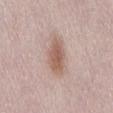notes — total-body-photography surveillance lesion; no biopsy
patient — female, aged 48–52
image-analysis metrics — a lesion area of about 11 mm², an eccentricity of roughly 0.9, and a symmetry-axis asymmetry near 0.15
lesion diameter — ≈5.5 mm
site — the back
image — ~15 mm tile from a whole-body skin photo